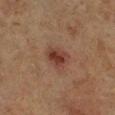<tbp_lesion>
  <biopsy_status>not biopsied; imaged during a skin examination</biopsy_status>
  <image>
    <source>total-body photography crop</source>
    <field_of_view_mm>15</field_of_view_mm>
  </image>
  <lesion_size>
    <long_diameter_mm_approx>3.0</long_diameter_mm_approx>
  </lesion_size>
  <lighting>cross-polarized</lighting>
  <patient>
    <sex>male</sex>
    <age_approx>85+</age_approx>
  </patient>
  <site>left lower leg</site>
</tbp_lesion>